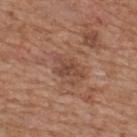Q: How was this image acquired?
A: ~15 mm crop, total-body skin-cancer survey
Q: How large is the lesion?
A: ~3.5 mm (longest diameter)
Q: Who is the patient?
A: male, aged approximately 65
Q: Automated lesion metrics?
A: an area of roughly 4.5 mm² and an outline eccentricity of about 0.8 (0 = round, 1 = elongated); a lesion color around L≈45 a*≈21 b*≈28 in CIELAB and roughly 8 lightness units darker than nearby skin
Q: Where on the body is the lesion?
A: the upper back
Q: What lighting was used for the tile?
A: white-light illumination